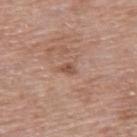• notes: imaged on a skin check; not biopsied
• tile lighting: white-light
• lesion size: ~3 mm (longest diameter)
• anatomic site: the upper back
• subject: female, roughly 60 years of age
• image: ~15 mm crop, total-body skin-cancer survey